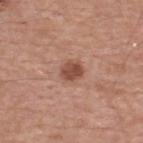Q: Was this lesion biopsied?
A: total-body-photography surveillance lesion; no biopsy
Q: Lesion location?
A: the back
Q: What is the lesion's diameter?
A: ≈2.5 mm
Q: What lighting was used for the tile?
A: white-light illumination
Q: Who is the patient?
A: male, in their mid- to late 50s
Q: What is the imaging modality?
A: ~15 mm tile from a whole-body skin photo
Q: Automated lesion metrics?
A: an area of roughly 4.5 mm², a shape eccentricity near 0.55, and two-axis asymmetry of about 0.2; roughly 12 lightness units darker than nearby skin and a normalized border contrast of about 8.5; a border-irregularity index near 1.5/10, a color-variation rating of about 2.5/10, and peripheral color asymmetry of about 1